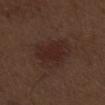Q: Is there a histopathology result?
A: total-body-photography surveillance lesion; no biopsy